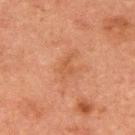Q: Was a biopsy performed?
A: total-body-photography surveillance lesion; no biopsy
Q: Patient demographics?
A: male, about 50 years old
Q: Lesion location?
A: the upper back
Q: What did automated image analysis measure?
A: a footprint of about 3.5 mm² and a shape-asymmetry score of about 0.4 (0 = symmetric); an average lesion color of about L≈43 a*≈21 b*≈31 (CIELAB) and a lesion–skin lightness drop of about 5; a border-irregularity rating of about 4/10, a within-lesion color-variation index near 1/10, and peripheral color asymmetry of about 0.5; a classifier nevus-likeness of about 0/100 and lesion-presence confidence of about 100/100
Q: What lighting was used for the tile?
A: cross-polarized
Q: What is the imaging modality?
A: total-body-photography crop, ~15 mm field of view
Q: Lesion size?
A: ≈2.5 mm